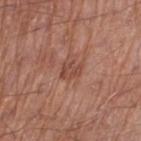follow-up: total-body-photography surveillance lesion; no biopsy | body site: the left thigh | lesion diameter: about 2.5 mm | illumination: white-light | patient: male, in their mid- to late 60s | imaging modality: ~15 mm tile from a whole-body skin photo | automated lesion analysis: an area of roughly 3.5 mm², an outline eccentricity of about 0.7 (0 = round, 1 = elongated), and a symmetry-axis asymmetry near 0.3; a lesion color around L≈47 a*≈24 b*≈28 in CIELAB, about 8 CIELAB-L* units darker than the surrounding skin, and a normalized lesion–skin contrast near 6; an automated nevus-likeness rating near 0 out of 100.